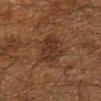Recorded during total-body skin imaging; not selected for excision or biopsy. A roughly 15 mm field-of-view crop from a total-body skin photograph. This is a cross-polarized tile. The total-body-photography lesion software estimated a footprint of about 10 mm² and a shape-asymmetry score of about 0.3 (0 = symmetric). It also reported an average lesion color of about L≈24 a*≈15 b*≈22 (CIELAB), roughly 6 lightness units darker than nearby skin, and a normalized lesion–skin contrast near 7. It also reported border irregularity of about 3.5 on a 0–10 scale, internal color variation of about 2.5 on a 0–10 scale, and peripheral color asymmetry of about 1. A male patient, roughly 60 years of age. Located on the left lower leg.